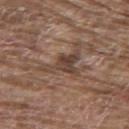The lesion was tiled from a total-body skin photograph and was not biopsied.
On the upper back.
Automated image analysis of the tile measured a lesion area of about 8 mm², an eccentricity of roughly 0.8, and a symmetry-axis asymmetry near 0.55. It also reported a lesion color around L≈42 a*≈16 b*≈24 in CIELAB, about 9 CIELAB-L* units darker than the surrounding skin, and a normalized lesion–skin contrast near 7.5. The software also gave a border-irregularity index near 7.5/10, a color-variation rating of about 3.5/10, and a peripheral color-asymmetry measure near 1. The analysis additionally found an automated nevus-likeness rating near 0 out of 100 and a lesion-detection confidence of about 55/100.
The subject is a male aged around 80.
A 15 mm close-up extracted from a 3D total-body photography capture.